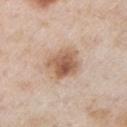Clinical impression:
Imaged during a routine full-body skin examination; the lesion was not biopsied and no histopathology is available.
Background:
From the arm. A male subject, roughly 55 years of age. Longest diameter approximately 4.5 mm. This is a white-light tile. The total-body-photography lesion software estimated a footprint of about 12 mm² and an eccentricity of roughly 0.65. The analysis additionally found border irregularity of about 2 on a 0–10 scale and a within-lesion color-variation index near 6.5/10. Cropped from a total-body skin-imaging series; the visible field is about 15 mm.